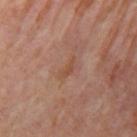Impression: This lesion was catalogued during total-body skin photography and was not selected for biopsy. Background: Captured under cross-polarized illumination. Located on the right upper arm. Longest diameter approximately 3 mm. A female subject aged approximately 50. A 15 mm close-up extracted from a 3D total-body photography capture.